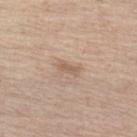Clinical impression:
This lesion was catalogued during total-body skin photography and was not selected for biopsy.
Clinical summary:
A male subject about 70 years old. On the left lower leg. Approximately 2.5 mm at its widest. Imaged with white-light lighting. A region of skin cropped from a whole-body photographic capture, roughly 15 mm wide.